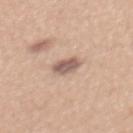{"biopsy_status": "not biopsied; imaged during a skin examination", "automated_metrics": {"nevus_likeness_0_100": 55, "lesion_detection_confidence_0_100": 100}, "image": {"source": "total-body photography crop", "field_of_view_mm": 15}, "lighting": "white-light", "lesion_size": {"long_diameter_mm_approx": 2.5}, "patient": {"sex": "female", "age_approx": 30}, "site": "upper back"}